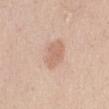biopsy status: no biopsy performed (imaged during a skin exam) | anatomic site: the chest | image source: ~15 mm crop, total-body skin-cancer survey | subject: female, aged 23 to 27 | size: about 4 mm | illumination: white-light.